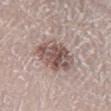The lesion was tiled from a total-body skin photograph and was not biopsied. A male subject, aged around 65. A lesion tile, about 15 mm wide, cut from a 3D total-body photograph. The lesion is on the leg.